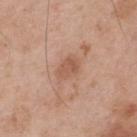follow-up: no biopsy performed (imaged during a skin exam); automated metrics: an outline eccentricity of about 0.7 (0 = round, 1 = elongated); patient: male, in their mid- to late 50s; diameter: ≈3 mm; anatomic site: the upper back; imaging modality: ~15 mm tile from a whole-body skin photo; lighting: white-light illumination.